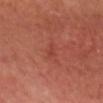| feature | finding |
|---|---|
| notes | imaged on a skin check; not biopsied |
| subject | male, about 65 years old |
| imaging modality | total-body-photography crop, ~15 mm field of view |
| lighting | cross-polarized |
| lesion diameter | about 2.5 mm |
| location | the head or neck |